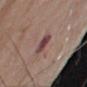Impression: No biopsy was performed on this lesion — it was imaged during a full skin examination and was not determined to be concerning. Acquisition and patient details: The patient is a male roughly 75 years of age. The lesion is located on the right upper arm. Imaged with white-light lighting. The recorded lesion diameter is about 3 mm. Cropped from a total-body skin-imaging series; the visible field is about 15 mm.